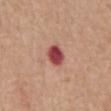imaging modality: ~15 mm crop, total-body skin-cancer survey | site: the abdomen | image-analysis metrics: an outline eccentricity of about 0.55 (0 = round, 1 = elongated) and a symmetry-axis asymmetry near 0.15; a within-lesion color-variation index near 4/10; a nevus-likeness score of about 0/100 and a lesion-detection confidence of about 100/100 | subject: male, approximately 65 years of age.